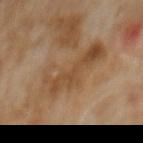Imaged during a routine full-body skin examination; the lesion was not biopsied and no histopathology is available.
A male subject, aged 58 to 62.
Measured at roughly 7.5 mm in maximum diameter.
Cropped from a total-body skin-imaging series; the visible field is about 15 mm.
Located on the abdomen.